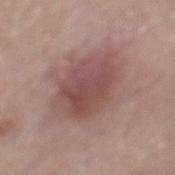Q: Was this lesion biopsied?
A: catalogued during a skin exam; not biopsied
Q: What are the patient's age and sex?
A: male, approximately 70 years of age
Q: Where on the body is the lesion?
A: the mid back
Q: What lighting was used for the tile?
A: white-light illumination
Q: What kind of image is this?
A: total-body-photography crop, ~15 mm field of view